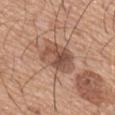| key | value |
|---|---|
| biopsy status | total-body-photography surveillance lesion; no biopsy |
| imaging modality | total-body-photography crop, ~15 mm field of view |
| size | about 5.5 mm |
| lighting | white-light illumination |
| body site | the mid back |
| subject | male, aged 63–67 |